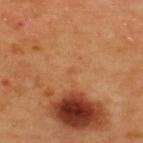follow-up: imaged on a skin check; not biopsied
acquisition: 15 mm crop, total-body photography
patient: female, aged 48–52
anatomic site: the upper back